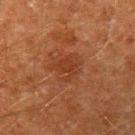Recorded during total-body skin imaging; not selected for excision or biopsy. A male subject roughly 60 years of age. Located on the arm. Imaged with cross-polarized lighting. A 15 mm close-up extracted from a 3D total-body photography capture.